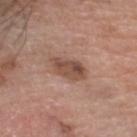workup = imaged on a skin check; not biopsied
lighting = white-light
size = ≈4 mm
imaging modality = ~15 mm tile from a whole-body skin photo
site = the head or neck
patient = male, approximately 60 years of age